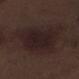notes=no biopsy performed (imaged during a skin exam); patient=male, aged 68–72; acquisition=15 mm crop, total-body photography; anatomic site=the left lower leg.